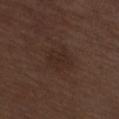biopsy status = imaged on a skin check; not biopsied
site = the right thigh
subject = male, about 70 years old
lighting = white-light illumination
size = ~3.5 mm (longest diameter)
image source = 15 mm crop, total-body photography